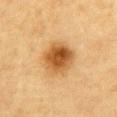follow-up: catalogued during a skin exam; not biopsied
automated metrics: a lesion color around L≈48 a*≈21 b*≈40 in CIELAB, roughly 13 lightness units darker than nearby skin, and a lesion-to-skin contrast of about 10 (normalized; higher = more distinct); border irregularity of about 1.5 on a 0–10 scale, internal color variation of about 6 on a 0–10 scale, and radial color variation of about 2; a nevus-likeness score of about 100/100 and a lesion-detection confidence of about 100/100
acquisition: ~15 mm crop, total-body skin-cancer survey
lesion diameter: about 4 mm
lighting: cross-polarized
patient: male, in their mid- to late 80s or older
site: the abdomen